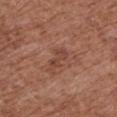Recorded during total-body skin imaging; not selected for excision or biopsy.
Automated tile analysis of the lesion measured an eccentricity of roughly 0.85. The analysis additionally found an average lesion color of about L≈44 a*≈23 b*≈28 (CIELAB), about 7 CIELAB-L* units darker than the surrounding skin, and a lesion-to-skin contrast of about 6 (normalized; higher = more distinct). The software also gave internal color variation of about 2 on a 0–10 scale and peripheral color asymmetry of about 1.
This image is a 15 mm lesion crop taken from a total-body photograph.
A female subject, in their mid- to late 70s.
This is a white-light tile.
On the chest.
The lesion's longest dimension is about 3 mm.